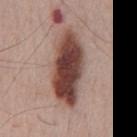Imaged during a routine full-body skin examination; the lesion was not biopsied and no histopathology is available.
Automated image analysis of the tile measured a lesion area of about 24 mm², an eccentricity of roughly 0.9, and a symmetry-axis asymmetry near 0.2. It also reported an automated nevus-likeness rating near 100 out of 100 and a lesion-detection confidence of about 100/100.
Longest diameter approximately 9 mm.
A male patient, aged 63 to 67.
A roughly 15 mm field-of-view crop from a total-body skin photograph.
Captured under white-light illumination.
The lesion is on the front of the torso.